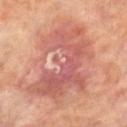Context: A 15 mm close-up extracted from a 3D total-body photography capture. The lesion is on the right lower leg. A female patient, in their mid-60s. Measured at roughly 9.5 mm in maximum diameter. Conclusion: Biopsy histopathology demonstrated a lesion of indeterminate malignant potential: actinic keratosis.